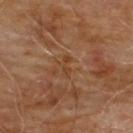Clinical impression:
Imaged during a routine full-body skin examination; the lesion was not biopsied and no histopathology is available.
Context:
Measured at roughly 2.5 mm in maximum diameter. Imaged with cross-polarized lighting. A roughly 15 mm field-of-view crop from a total-body skin photograph. A male subject, aged 58–62. The lesion is on the chest.